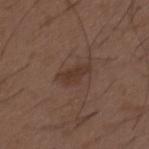Q: Is there a histopathology result?
A: total-body-photography surveillance lesion; no biopsy
Q: How was the tile lit?
A: white-light illumination
Q: Patient demographics?
A: male, about 50 years old
Q: What is the imaging modality?
A: total-body-photography crop, ~15 mm field of view
Q: Where on the body is the lesion?
A: the upper back
Q: How large is the lesion?
A: ≈4 mm